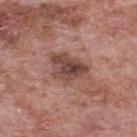Case summary:
* notes: total-body-photography surveillance lesion; no biopsy
* patient: male, aged 68–72
* location: the mid back
* image source: ~15 mm tile from a whole-body skin photo
* automated lesion analysis: an average lesion color of about L≈46 a*≈21 b*≈24 (CIELAB) and a lesion–skin lightness drop of about 12; a border-irregularity rating of about 3.5/10 and a within-lesion color-variation index near 6.5/10; lesion-presence confidence of about 100/100
* illumination: white-light illumination
* size: ~5 mm (longest diameter)